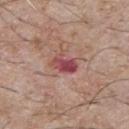Q: Is there a histopathology result?
A: no biopsy performed (imaged during a skin exam)
Q: What did automated image analysis measure?
A: an area of roughly 5.5 mm², an eccentricity of roughly 0.8, and a shape-asymmetry score of about 0.3 (0 = symmetric); a mean CIELAB color near L≈47 a*≈29 b*≈21 and roughly 12 lightness units darker than nearby skin; a within-lesion color-variation index near 6/10 and peripheral color asymmetry of about 2; an automated nevus-likeness rating near 0 out of 100 and a detector confidence of about 100 out of 100 that the crop contains a lesion
Q: Where on the body is the lesion?
A: the chest
Q: How was this image acquired?
A: 15 mm crop, total-body photography
Q: Lesion size?
A: ~3 mm (longest diameter)
Q: Patient demographics?
A: male, aged approximately 65